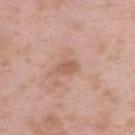{"biopsy_status": "not biopsied; imaged during a skin examination", "patient": {"sex": "male", "age_approx": 55}, "image": {"source": "total-body photography crop", "field_of_view_mm": 15}, "site": "back"}